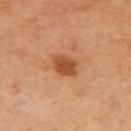The lesion was tiled from a total-body skin photograph and was not biopsied.
Imaged with cross-polarized lighting.
The lesion is located on the right upper arm.
The subject is a male aged 58 to 62.
About 2.5 mm across.
An algorithmic analysis of the crop reported a mean CIELAB color near L≈48 a*≈26 b*≈37 and roughly 12 lightness units darker than nearby skin.
Cropped from a whole-body photographic skin survey; the tile spans about 15 mm.